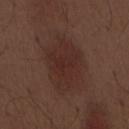Impression:
Captured during whole-body skin photography for melanoma surveillance; the lesion was not biopsied.
Clinical summary:
An algorithmic analysis of the crop reported an automated nevus-likeness rating near 55 out of 100 and a lesion-detection confidence of about 100/100. This is a white-light tile. From the abdomen. Approximately 7 mm at its widest. A region of skin cropped from a whole-body photographic capture, roughly 15 mm wide. A male subject in their 70s.